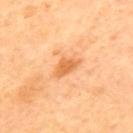Part of a total-body skin-imaging series; this lesion was reviewed on a skin check and was not flagged for biopsy. Automated image analysis of the tile measured a lesion area of about 5.5 mm² and a shape-asymmetry score of about 0.35 (0 = symmetric). It also reported an average lesion color of about L≈66 a*≈27 b*≈46 (CIELAB), a lesion–skin lightness drop of about 10, and a normalized border contrast of about 7. The software also gave a detector confidence of about 100 out of 100 that the crop contains a lesion. Captured under cross-polarized illumination. About 3.5 mm across. On the upper back. Cropped from a total-body skin-imaging series; the visible field is about 15 mm. A female patient, aged around 50.